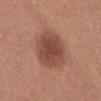- notes: catalogued during a skin exam; not biopsied
- patient: female, aged 23 to 27
- acquisition: ~15 mm tile from a whole-body skin photo
- lesion size: about 5.5 mm
- anatomic site: the front of the torso
- image-analysis metrics: an outline eccentricity of about 0.65 (0 = round, 1 = elongated) and a shape-asymmetry score of about 0.1 (0 = symmetric)